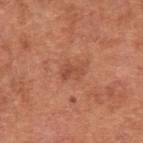Notes:
* biopsy status — imaged on a skin check; not biopsied